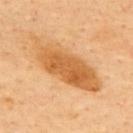Q: Was a biopsy performed?
A: catalogued during a skin exam; not biopsied
Q: Lesion size?
A: ~10 mm (longest diameter)
Q: Patient demographics?
A: male, approximately 65 years of age
Q: How was the tile lit?
A: cross-polarized illumination
Q: What did automated image analysis measure?
A: border irregularity of about 3.5 on a 0–10 scale, a within-lesion color-variation index near 4.5/10, and peripheral color asymmetry of about 1.5
Q: What is the imaging modality?
A: ~15 mm tile from a whole-body skin photo
Q: Where on the body is the lesion?
A: the upper back